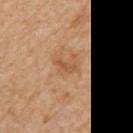The lesion was photographed on a routine skin check and not biopsied; there is no pathology result.